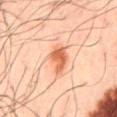Recorded during total-body skin imaging; not selected for excision or biopsy.
A 15 mm crop from a total-body photograph taken for skin-cancer surveillance.
The lesion is located on the mid back.
Measured at roughly 4.5 mm in maximum diameter.
The tile uses cross-polarized illumination.
A male patient in their 50s.
The lesion-visualizer software estimated a shape eccentricity near 0.85 and a symmetry-axis asymmetry near 0.25.